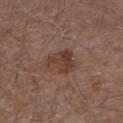Case summary:
* follow-up: catalogued during a skin exam; not biopsied
* TBP lesion metrics: an area of roughly 8 mm² and a symmetry-axis asymmetry near 0.35; roughly 8 lightness units darker than nearby skin and a lesion-to-skin contrast of about 7.5 (normalized; higher = more distinct); a border-irregularity rating of about 4/10, internal color variation of about 3.5 on a 0–10 scale, and a peripheral color-asymmetry measure near 1
* location: the right upper arm
* patient: male, aged 53 to 57
* image: total-body-photography crop, ~15 mm field of view
* lighting: white-light illumination
* size: about 3.5 mm